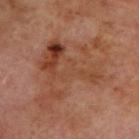Clinical summary: A male subject in their 70s. From the upper back. A 15 mm crop from a total-body photograph taken for skin-cancer surveillance. About 10 mm across. This is a cross-polarized tile.